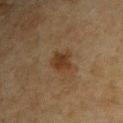Q: What is the anatomic site?
A: the left upper arm
Q: What lighting was used for the tile?
A: cross-polarized illumination
Q: Patient demographics?
A: male, in their mid- to late 60s
Q: What kind of image is this?
A: ~15 mm crop, total-body skin-cancer survey
Q: Lesion size?
A: ~3.5 mm (longest diameter)